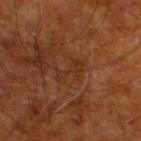<record>
<biopsy_status>not biopsied; imaged during a skin examination</biopsy_status>
<site>left lower leg</site>
<image>
  <source>total-body photography crop</source>
  <field_of_view_mm>15</field_of_view_mm>
</image>
<patient>
  <sex>male</sex>
  <age_approx>80</age_approx>
</patient>
</record>